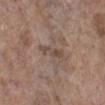No biopsy was performed on this lesion — it was imaged during a full skin examination and was not determined to be concerning. A female patient aged around 85 or older. A 15 mm close-up tile from a total-body photography series done for melanoma screening. The lesion is on the left lower leg. The total-body-photography lesion software estimated an outline eccentricity of about 0.95 (0 = round, 1 = elongated). The analysis additionally found a lesion color around L≈47 a*≈15 b*≈23 in CIELAB, a lesion–skin lightness drop of about 8, and a normalized lesion–skin contrast near 6. And it measured border irregularity of about 5.5 on a 0–10 scale, a color-variation rating of about 0.5/10, and radial color variation of about 0. The software also gave an automated nevus-likeness rating near 0 out of 100.